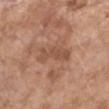Impression: This lesion was catalogued during total-body skin photography and was not selected for biopsy. Clinical summary: On the right upper arm. A female patient, about 85 years old or older. Cropped from a whole-body photographic skin survey; the tile spans about 15 mm.